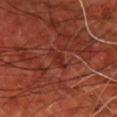workup: catalogued during a skin exam; not biopsied
subject: male, in their 60s
acquisition: 15 mm crop, total-body photography
body site: the chest